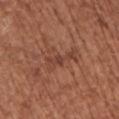- biopsy status — total-body-photography surveillance lesion; no biopsy
- image — ~15 mm crop, total-body skin-cancer survey
- patient — female, approximately 75 years of age
- tile lighting — white-light illumination
- anatomic site — the left upper arm